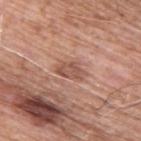Clinical impression: Imaged during a routine full-body skin examination; the lesion was not biopsied and no histopathology is available. Context: A male patient approximately 60 years of age. Longest diameter approximately 3 mm. A 15 mm close-up tile from a total-body photography series done for melanoma screening. Automated tile analysis of the lesion measured a lesion color around L≈53 a*≈22 b*≈28 in CIELAB and a lesion-to-skin contrast of about 6.5 (normalized; higher = more distinct). It also reported a border-irregularity index near 3.5/10. The software also gave a classifier nevus-likeness of about 0/100. Located on the upper back. Imaged with white-light lighting.